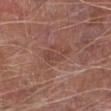| feature | finding |
|---|---|
| workup | catalogued during a skin exam; not biopsied |
| imaging modality | ~15 mm crop, total-body skin-cancer survey |
| illumination | white-light illumination |
| automated lesion analysis | a lesion color around L≈44 a*≈22 b*≈25 in CIELAB, roughly 6 lightness units darker than nearby skin, and a lesion-to-skin contrast of about 5 (normalized; higher = more distinct); border irregularity of about 6 on a 0–10 scale, a within-lesion color-variation index near 1/10, and a peripheral color-asymmetry measure near 0.5 |
| subject | male, aged approximately 65 |
| site | the right lower leg |